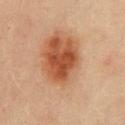The lesion was photographed on a routine skin check and not biopsied; there is no pathology result. A female subject aged around 35. The lesion is located on the mid back. A close-up tile cropped from a whole-body skin photograph, about 15 mm across.